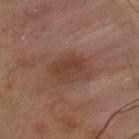Case summary:
– site · the upper back
– image source · ~15 mm crop, total-body skin-cancer survey
– patient · male, about 40 years old